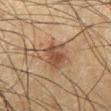| feature | finding |
|---|---|
| image source | ~15 mm crop, total-body skin-cancer survey |
| anatomic site | the right lower leg |
| subject | male, aged around 35 |
| lighting | cross-polarized illumination |
| automated lesion analysis | a footprint of about 7 mm²; roughly 10 lightness units darker than nearby skin and a normalized border contrast of about 8 |
| diameter | about 3.5 mm |